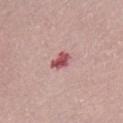The lesion was photographed on a routine skin check and not biopsied; there is no pathology result. From the chest. A 15 mm close-up tile from a total-body photography series done for melanoma screening. The patient is a female aged 63 to 67. Longest diameter approximately 3 mm.